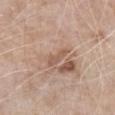Captured during whole-body skin photography for melanoma surveillance; the lesion was not biopsied. Located on the chest. A male subject aged approximately 80. A 15 mm close-up extracted from a 3D total-body photography capture. The tile uses white-light illumination. Automated image analysis of the tile measured a footprint of about 3 mm² and a shape-asymmetry score of about 0.4 (0 = symmetric). And it measured an average lesion color of about L≈56 a*≈19 b*≈27 (CIELAB), about 8 CIELAB-L* units darker than the surrounding skin, and a normalized border contrast of about 5.5. And it measured a border-irregularity rating of about 4.5/10, a color-variation rating of about 0/10, and radial color variation of about 0. And it measured an automated nevus-likeness rating near 0 out of 100 and a lesion-detection confidence of about 95/100.